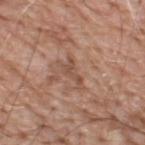Imaged during a routine full-body skin examination; the lesion was not biopsied and no histopathology is available. A 15 mm close-up tile from a total-body photography series done for melanoma screening. Located on the upper back. A male patient, in their 60s.